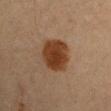Impression:
The lesion was photographed on a routine skin check and not biopsied; there is no pathology result.
Image and clinical context:
Measured at roughly 4.5 mm in maximum diameter. A close-up tile cropped from a whole-body skin photograph, about 15 mm across. Imaged with cross-polarized lighting. A male subject aged 58–62. The lesion-visualizer software estimated a footprint of about 13 mm², a shape eccentricity near 0.65, and two-axis asymmetry of about 0.1. It also reported an automated nevus-likeness rating near 100 out of 100 and a detector confidence of about 100 out of 100 that the crop contains a lesion. The lesion is located on the right upper arm.